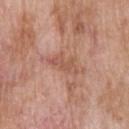Recorded during total-body skin imaging; not selected for excision or biopsy. This is a white-light tile. Measured at roughly 3.5 mm in maximum diameter. The subject is a male about 60 years old. The lesion is located on the upper back. A 15 mm crop from a total-body photograph taken for skin-cancer surveillance.